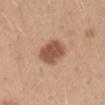| field | value |
|---|---|
| body site | the right upper arm |
| lesion diameter | ~4 mm (longest diameter) |
| illumination | white-light |
| subject | male, aged around 30 |
| imaging modality | ~15 mm tile from a whole-body skin photo |